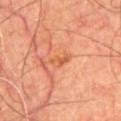<tbp_lesion>
<biopsy_status>not biopsied; imaged during a skin examination</biopsy_status>
</tbp_lesion>